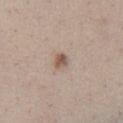{"biopsy_status": "not biopsied; imaged during a skin examination", "image": {"source": "total-body photography crop", "field_of_view_mm": 15}, "patient": {"sex": "male", "age_approx": 30}, "site": "chest", "lighting": "white-light", "automated_metrics": {"area_mm2_approx": 3.0, "eccentricity": 0.55, "shape_asymmetry": 0.3, "cielab_L": 55, "cielab_a": 16, "cielab_b": 26, "vs_skin_darker_L": 12.0, "vs_skin_contrast_norm": 8.0, "peripheral_color_asymmetry": 1.5, "nevus_likeness_0_100": 90, "lesion_detection_confidence_0_100": 100}, "lesion_size": {"long_diameter_mm_approx": 2.0}}